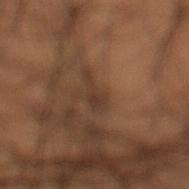Impression: The lesion was photographed on a routine skin check and not biopsied; there is no pathology result. Context: Cropped from a whole-body photographic skin survey; the tile spans about 15 mm. The total-body-photography lesion software estimated a border-irregularity rating of about 4.5/10, a within-lesion color-variation index near 1/10, and radial color variation of about 0.5. Imaged with cross-polarized lighting. A male subject, approximately 50 years of age. Located on the left lower leg. Measured at roughly 3 mm in maximum diameter.